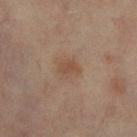Clinical impression: Imaged during a routine full-body skin examination; the lesion was not biopsied and no histopathology is available. Acquisition and patient details: Automated image analysis of the tile measured a footprint of about 4 mm² and an eccentricity of roughly 0.65. The analysis additionally found border irregularity of about 2.5 on a 0–10 scale, internal color variation of about 1.5 on a 0–10 scale, and peripheral color asymmetry of about 0.5. It also reported a classifier nevus-likeness of about 70/100. Located on the leg. Captured under cross-polarized illumination. A female patient, roughly 65 years of age. A region of skin cropped from a whole-body photographic capture, roughly 15 mm wide.